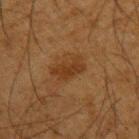A male patient roughly 65 years of age. Automated image analysis of the tile measured a footprint of about 6 mm², a shape eccentricity near 0.85, and a shape-asymmetry score of about 0.35 (0 = symmetric). And it measured a lesion color around L≈29 a*≈18 b*≈30 in CIELAB. The analysis additionally found a classifier nevus-likeness of about 20/100 and a detector confidence of about 100 out of 100 that the crop contains a lesion. Longest diameter approximately 4 mm. A close-up tile cropped from a whole-body skin photograph, about 15 mm across. From the back. Imaged with cross-polarized lighting.